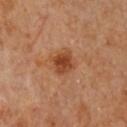- workup · imaged on a skin check; not biopsied
- patient · female
- illumination · cross-polarized
- body site · the chest
- image · ~15 mm tile from a whole-body skin photo